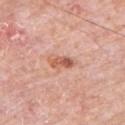Impression: Recorded during total-body skin imaging; not selected for excision or biopsy. Background: On the left upper arm. Automated tile analysis of the lesion measured a footprint of about 4.5 mm², an eccentricity of roughly 0.9, and a symmetry-axis asymmetry near 0.35. It also reported a mean CIELAB color near L≈59 a*≈26 b*≈32 and a normalized lesion–skin contrast near 7.5. The software also gave a border-irregularity rating of about 3.5/10, a within-lesion color-variation index near 6/10, and peripheral color asymmetry of about 2.5. The software also gave a nevus-likeness score of about 85/100. Imaged with white-light lighting. A male patient aged around 80. Longest diameter approximately 3.5 mm. A region of skin cropped from a whole-body photographic capture, roughly 15 mm wide.